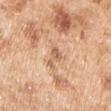Captured during whole-body skin photography for melanoma surveillance; the lesion was not biopsied. Imaged with white-light lighting. Approximately 3 mm at its widest. A lesion tile, about 15 mm wide, cut from a 3D total-body photograph. A male subject in their mid- to late 50s. Automated tile analysis of the lesion measured a mean CIELAB color near L≈63 a*≈21 b*≈34 and a normalized lesion–skin contrast near 6. The analysis additionally found a border-irregularity rating of about 5.5/10 and radial color variation of about 0.5. The software also gave a classifier nevus-likeness of about 0/100 and a lesion-detection confidence of about 100/100. The lesion is on the left upper arm.